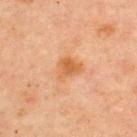No biopsy was performed on this lesion — it was imaged during a full skin examination and was not determined to be concerning. The lesion is located on the upper back. Cropped from a whole-body photographic skin survey; the tile spans about 15 mm. The patient is a male in their 60s. The lesion-visualizer software estimated a lesion color around L≈51 a*≈22 b*≈36 in CIELAB, about 8 CIELAB-L* units darker than the surrounding skin, and a normalized lesion–skin contrast near 7. The recorded lesion diameter is about 3 mm.